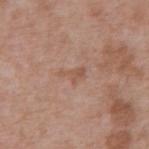<lesion>
<biopsy_status>not biopsied; imaged during a skin examination</biopsy_status>
<lighting>white-light</lighting>
<site>front of the torso</site>
<image>
  <source>total-body photography crop</source>
  <field_of_view_mm>15</field_of_view_mm>
</image>
<patient>
  <sex>male</sex>
  <age_approx>70</age_approx>
</patient>
</lesion>